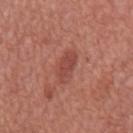Impression:
Imaged during a routine full-body skin examination; the lesion was not biopsied and no histopathology is available.
Background:
The lesion is located on the back. The patient is a male aged approximately 65. A roughly 15 mm field-of-view crop from a total-body skin photograph.